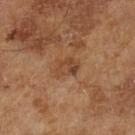follow-up: no biopsy performed (imaged during a skin exam)